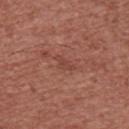| field | value |
|---|---|
| notes | total-body-photography surveillance lesion; no biopsy |
| automated metrics | a footprint of about 2.5 mm², an outline eccentricity of about 0.85 (0 = round, 1 = elongated), and a shape-asymmetry score of about 0.5 (0 = symmetric) |
| size | about 2.5 mm |
| site | the chest |
| subject | male, in their mid-40s |
| lighting | white-light illumination |
| acquisition | ~15 mm crop, total-body skin-cancer survey |